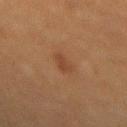A 15 mm close-up tile from a total-body photography series done for melanoma screening.
The patient is a male approximately 50 years of age.
Captured under cross-polarized illumination.
The lesion's longest dimension is about 3 mm.
On the mid back.